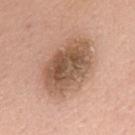Imaged during a routine full-body skin examination; the lesion was not biopsied and no histopathology is available. The total-body-photography lesion software estimated roughly 14 lightness units darker than nearby skin and a normalized lesion–skin contrast near 9. It also reported internal color variation of about 6 on a 0–10 scale and a peripheral color-asymmetry measure near 2. And it measured a nevus-likeness score of about 5/100 and a lesion-detection confidence of about 100/100. A 15 mm crop from a total-body photograph taken for skin-cancer surveillance. The lesion's longest dimension is about 7.5 mm. From the mid back. A male patient, aged around 55.